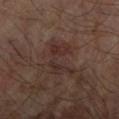The tile uses cross-polarized illumination. Cropped from a total-body skin-imaging series; the visible field is about 15 mm. Automated image analysis of the tile measured border irregularity of about 8.5 on a 0–10 scale, a color-variation rating of about 4/10, and a peripheral color-asymmetry measure near 1.5. The analysis additionally found a nevus-likeness score of about 0/100 and a lesion-detection confidence of about 95/100. Approximately 5 mm at its widest. A male patient about 65 years old. The lesion is on the right forearm.